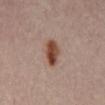Imaged during a routine full-body skin examination; the lesion was not biopsied and no histopathology is available. A patient roughly 55 years of age. An algorithmic analysis of the crop reported an area of roughly 7 mm², a shape eccentricity near 0.85, and two-axis asymmetry of about 0.25. It also reported an average lesion color of about L≈45 a*≈20 b*≈27 (CIELAB), about 13 CIELAB-L* units darker than the surrounding skin, and a normalized lesion–skin contrast near 11. Cropped from a total-body skin-imaging series; the visible field is about 15 mm. On the abdomen. Approximately 4 mm at its widest. Imaged with cross-polarized lighting.